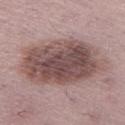• notes: total-body-photography surveillance lesion; no biopsy